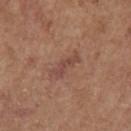image source=~15 mm tile from a whole-body skin photo
patient=female, in their mid- to late 60s
location=the left upper arm
size=about 4 mm